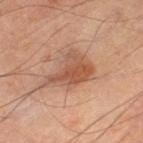biopsy status: no biopsy performed (imaged during a skin exam)
lighting: cross-polarized illumination
anatomic site: the left thigh
image source: 15 mm crop, total-body photography
image-analysis metrics: an area of roughly 13 mm² and an outline eccentricity of about 0.75 (0 = round, 1 = elongated); an average lesion color of about L≈52 a*≈22 b*≈31 (CIELAB) and a lesion-to-skin contrast of about 7 (normalized; higher = more distinct); border irregularity of about 5 on a 0–10 scale and peripheral color asymmetry of about 1.5; lesion-presence confidence of about 100/100
subject: male, roughly 65 years of age